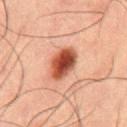The lesion was photographed on a routine skin check and not biopsied; there is no pathology result. Located on the abdomen. A 15 mm close-up tile from a total-body photography series done for melanoma screening. A male subject, about 50 years old. This is a cross-polarized tile.